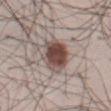The lesion was tiled from a total-body skin photograph and was not biopsied. This image is a 15 mm lesion crop taken from a total-body photograph. The lesion is on the abdomen. The patient is a male approximately 35 years of age.